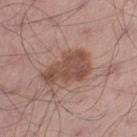This lesion was catalogued during total-body skin photography and was not selected for biopsy. Captured under white-light illumination. The subject is a male approximately 55 years of age. The recorded lesion diameter is about 5.5 mm. The lesion is on the leg. Automated tile analysis of the lesion measured a shape eccentricity near 0.85 and two-axis asymmetry of about 0.35. It also reported a lesion color around L≈49 a*≈20 b*≈25 in CIELAB and roughly 11 lightness units darker than nearby skin. And it measured a within-lesion color-variation index near 3/10 and a peripheral color-asymmetry measure near 1. And it measured lesion-presence confidence of about 100/100. A close-up tile cropped from a whole-body skin photograph, about 15 mm across.